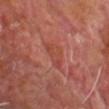Impression:
This lesion was catalogued during total-body skin photography and was not selected for biopsy.
Context:
A male subject, approximately 60 years of age. Cropped from a whole-body photographic skin survey; the tile spans about 15 mm. An algorithmic analysis of the crop reported a footprint of about 5.5 mm², an eccentricity of roughly 0.85, and two-axis asymmetry of about 0.4. The software also gave a mean CIELAB color near L≈44 a*≈28 b*≈29, roughly 5 lightness units darker than nearby skin, and a lesion-to-skin contrast of about 5 (normalized; higher = more distinct). The software also gave a border-irregularity rating of about 4/10, a within-lesion color-variation index near 2.5/10, and a peripheral color-asymmetry measure near 1. The analysis additionally found an automated nevus-likeness rating near 0 out of 100. About 3.5 mm across. The lesion is located on the back.